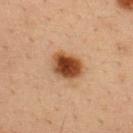This lesion was catalogued during total-body skin photography and was not selected for biopsy.
This image is a 15 mm lesion crop taken from a total-body photograph.
A male subject aged 33 to 37.
Captured under cross-polarized illumination.
About 4 mm across.
The lesion is located on the back.